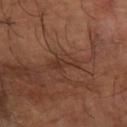The lesion was tiled from a total-body skin photograph and was not biopsied. About 4 mm across. A roughly 15 mm field-of-view crop from a total-body skin photograph. A male patient, aged 63 to 67. The lesion is located on the right forearm. Automated tile analysis of the lesion measured a footprint of about 5.5 mm². It also reported a lesion color around L≈34 a*≈20 b*≈26 in CIELAB, a lesion–skin lightness drop of about 6, and a lesion-to-skin contrast of about 5.5 (normalized; higher = more distinct). And it measured a border-irregularity rating of about 4.5/10 and a within-lesion color-variation index near 3/10.